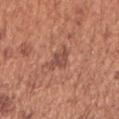Recorded during total-body skin imaging; not selected for excision or biopsy. A female patient, aged approximately 50. Cropped from a total-body skin-imaging series; the visible field is about 15 mm. Located on the right upper arm.